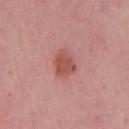Case summary:
• follow-up · imaged on a skin check; not biopsied
• imaging modality · total-body-photography crop, ~15 mm field of view
• patient · male, aged approximately 65
• site · the mid back